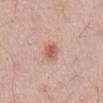Part of a total-body skin-imaging series; this lesion was reviewed on a skin check and was not flagged for biopsy. A male patient, in their mid- to late 50s. An algorithmic analysis of the crop reported an area of roughly 4 mm², an eccentricity of roughly 0.7, and two-axis asymmetry of about 0.2. The software also gave a mean CIELAB color near L≈59 a*≈21 b*≈28. Imaged with white-light lighting. The lesion is on the abdomen. A 15 mm close-up extracted from a 3D total-body photography capture. Approximately 2.5 mm at its widest.